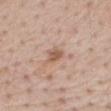The lesion was photographed on a routine skin check and not biopsied; there is no pathology result.
A close-up tile cropped from a whole-body skin photograph, about 15 mm across.
An algorithmic analysis of the crop reported a shape eccentricity near 0.75 and two-axis asymmetry of about 0.35. It also reported a lesion color around L≈57 a*≈19 b*≈30 in CIELAB and about 10 CIELAB-L* units darker than the surrounding skin. And it measured border irregularity of about 3 on a 0–10 scale, a within-lesion color-variation index near 2/10, and radial color variation of about 0.5. And it measured a nevus-likeness score of about 0/100 and a lesion-detection confidence of about 100/100.
The subject is a male in their mid-60s.
Captured under white-light illumination.
From the mid back.